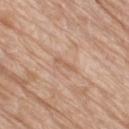{
  "biopsy_status": "not biopsied; imaged during a skin examination",
  "image": {
    "source": "total-body photography crop",
    "field_of_view_mm": 15
  },
  "lesion_size": {
    "long_diameter_mm_approx": 2.5
  },
  "automated_metrics": {
    "eccentricity": 0.95,
    "shape_asymmetry": 0.35,
    "color_variation_0_10": 0.0,
    "nevus_likeness_0_100": 0,
    "lesion_detection_confidence_0_100": 95
  },
  "site": "right thigh",
  "patient": {
    "sex": "female",
    "age_approx": 75
  }
}